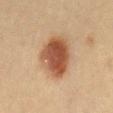  biopsy_status: not biopsied; imaged during a skin examination
  image:
    source: total-body photography crop
    field_of_view_mm: 15
  site: mid back
  patient:
    sex: female
    age_approx: 40
  lesion_size:
    long_diameter_mm_approx: 6.0
  automated_metrics:
    area_mm2_approx: 19.0
    vs_skin_darker_L: 13.0
    border_irregularity_0_10: 2.0
    peripheral_color_asymmetry: 1.5
  lighting: cross-polarized